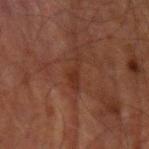workup: no biopsy performed (imaged during a skin exam)
location: the left upper arm
imaging modality: 15 mm crop, total-body photography
lighting: cross-polarized illumination
patient: male, aged approximately 70
TBP lesion metrics: a mean CIELAB color near L≈25 a*≈20 b*≈23, roughly 5 lightness units darker than nearby skin, and a normalized lesion–skin contrast near 6; a classifier nevus-likeness of about 0/100 and a detector confidence of about 85 out of 100 that the crop contains a lesion
size: ≈4 mm